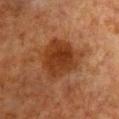Assessment:
Captured during whole-body skin photography for melanoma surveillance; the lesion was not biopsied.
Background:
On the chest. A roughly 15 mm field-of-view crop from a total-body skin photograph. Captured under cross-polarized illumination. A female patient, aged around 55.